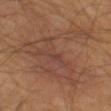The lesion was tiled from a total-body skin photograph and was not biopsied. Imaged with cross-polarized lighting. The lesion is on the left thigh. Measured at roughly 11 mm in maximum diameter. A roughly 15 mm field-of-view crop from a total-body skin photograph. The patient is a male aged 58–62.